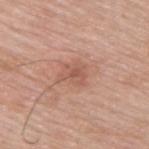workup — catalogued during a skin exam; not biopsied
patient — male, aged approximately 55
image — total-body-photography crop, ~15 mm field of view
anatomic site — the upper back